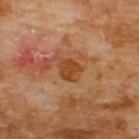{
  "biopsy_status": "not biopsied; imaged during a skin examination",
  "patient": {
    "sex": "male",
    "age_approx": 60
  },
  "image": {
    "source": "total-body photography crop",
    "field_of_view_mm": 15
  },
  "lesion_size": {
    "long_diameter_mm_approx": 3.0
  },
  "lighting": "cross-polarized",
  "automated_metrics": {
    "nevus_likeness_0_100": 5,
    "lesion_detection_confidence_0_100": 100
  },
  "site": "chest"
}